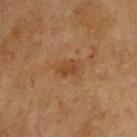<record>
  <lesion_size>
    <long_diameter_mm_approx>2.5</long_diameter_mm_approx>
  </lesion_size>
  <lighting>cross-polarized</lighting>
  <patient>
    <sex>male</sex>
    <age_approx>65</age_approx>
  </patient>
  <site>front of the torso</site>
  <image>
    <source>total-body photography crop</source>
    <field_of_view_mm>15</field_of_view_mm>
  </image>
</record>